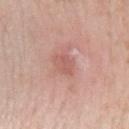Case summary:
* follow-up — no biopsy performed (imaged during a skin exam)
* subject — female, aged around 70
* location — the left forearm
* image source — 15 mm crop, total-body photography
* illumination — white-light illumination